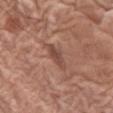<lesion>
<biopsy_status>not biopsied; imaged during a skin examination</biopsy_status>
<automated_metrics>
  <cielab_L>47</cielab_L>
  <cielab_a>22</cielab_a>
  <cielab_b>26</cielab_b>
  <vs_skin_darker_L>9.0</vs_skin_darker_L>
  <vs_skin_contrast_norm>7.0</vs_skin_contrast_norm>
</automated_metrics>
<lesion_size>
  <long_diameter_mm_approx>3.5</long_diameter_mm_approx>
</lesion_size>
<image>
  <source>total-body photography crop</source>
  <field_of_view_mm>15</field_of_view_mm>
</image>
<patient>
  <sex>female</sex>
  <age_approx>75</age_approx>
</patient>
<site>left thigh</site>
</lesion>